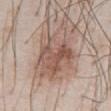No biopsy was performed on this lesion — it was imaged during a full skin examination and was not determined to be concerning. Cropped from a whole-body photographic skin survey; the tile spans about 15 mm. The subject is a male aged approximately 55. On the chest.